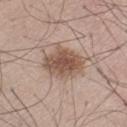The lesion was photographed on a routine skin check and not biopsied; there is no pathology result. The total-body-photography lesion software estimated a footprint of about 13 mm² and a shape-asymmetry score of about 0.15 (0 = symmetric). The software also gave an average lesion color of about L≈52 a*≈17 b*≈26 (CIELAB), about 13 CIELAB-L* units darker than the surrounding skin, and a lesion-to-skin contrast of about 9 (normalized; higher = more distinct). It also reported a border-irregularity index near 2/10, a color-variation rating of about 3.5/10, and radial color variation of about 1. And it measured an automated nevus-likeness rating near 95 out of 100 and lesion-presence confidence of about 100/100. A male subject roughly 45 years of age. The lesion is on the left thigh. Imaged with white-light lighting. Cropped from a total-body skin-imaging series; the visible field is about 15 mm.